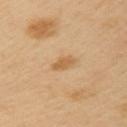Impression:
Captured during whole-body skin photography for melanoma surveillance; the lesion was not biopsied.
Context:
Cropped from a total-body skin-imaging series; the visible field is about 15 mm. A male patient aged 38–42. On the arm. The lesion-visualizer software estimated an outline eccentricity of about 0.85 (0 = round, 1 = elongated) and a shape-asymmetry score of about 0.25 (0 = symmetric). The software also gave an average lesion color of about L≈59 a*≈19 b*≈40 (CIELAB) and roughly 9 lightness units darker than nearby skin. It also reported a border-irregularity rating of about 2.5/10, a within-lesion color-variation index near 1/10, and radial color variation of about 0.5. It also reported a classifier nevus-likeness of about 65/100 and a lesion-detection confidence of about 100/100.